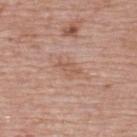No biopsy was performed on this lesion — it was imaged during a full skin examination and was not determined to be concerning. Approximately 3 mm at its widest. A female patient roughly 65 years of age. This image is a 15 mm lesion crop taken from a total-body photograph. This is a white-light tile. The total-body-photography lesion software estimated an average lesion color of about L≈57 a*≈20 b*≈29 (CIELAB), roughly 7 lightness units darker than nearby skin, and a normalized border contrast of about 5. Located on the upper back.